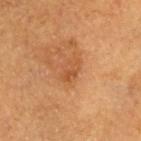The lesion was tiled from a total-body skin photograph and was not biopsied. From the head or neck. Cropped from a total-body skin-imaging series; the visible field is about 15 mm. Approximately 3 mm at its widest. The patient is a male roughly 85 years of age or older. Automated tile analysis of the lesion measured an area of roughly 4 mm², a shape eccentricity near 0.8, and a symmetry-axis asymmetry near 0.2. And it measured border irregularity of about 2.5 on a 0–10 scale, a within-lesion color-variation index near 3.5/10, and radial color variation of about 1.5. This is a cross-polarized tile.